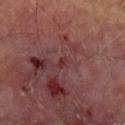Clinical impression:
Part of a total-body skin-imaging series; this lesion was reviewed on a skin check and was not flagged for biopsy.